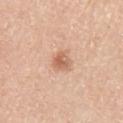{
  "lighting": "white-light",
  "image": {
    "source": "total-body photography crop",
    "field_of_view_mm": 15
  },
  "site": "arm",
  "patient": {
    "sex": "male",
    "age_approx": 60
  }
}